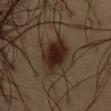Q: Is there a histopathology result?
A: imaged on a skin check; not biopsied
Q: What are the patient's age and sex?
A: male, aged approximately 50
Q: What kind of image is this?
A: ~15 mm crop, total-body skin-cancer survey
Q: What lighting was used for the tile?
A: cross-polarized illumination
Q: What is the lesion's diameter?
A: about 5 mm
Q: What is the anatomic site?
A: the chest